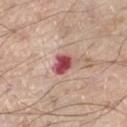No biopsy was performed on this lesion — it was imaged during a full skin examination and was not determined to be concerning.
Measured at roughly 3 mm in maximum diameter.
A male subject aged around 70.
The tile uses white-light illumination.
From the right thigh.
The total-body-photography lesion software estimated a lesion area of about 5.5 mm². The software also gave a border-irregularity rating of about 2/10, a within-lesion color-variation index near 5.5/10, and radial color variation of about 1.5. The software also gave a classifier nevus-likeness of about 0/100.
A 15 mm close-up tile from a total-body photography series done for melanoma screening.